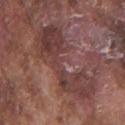Automated tile analysis of the lesion measured a footprint of about 28 mm², an outline eccentricity of about 0.9 (0 = round, 1 = elongated), and a shape-asymmetry score of about 0.45 (0 = symmetric). And it measured border irregularity of about 8.5 on a 0–10 scale, a color-variation rating of about 5/10, and a peripheral color-asymmetry measure near 1.5. The analysis additionally found a classifier nevus-likeness of about 0/100 and a detector confidence of about 60 out of 100 that the crop contains a lesion.
A 15 mm crop from a total-body photograph taken for skin-cancer surveillance.
A male patient, approximately 75 years of age.
This is a white-light tile.
The lesion is on the right upper arm.